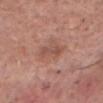Clinical impression: Imaged during a routine full-body skin examination; the lesion was not biopsied and no histopathology is available. Context: On the chest. The recorded lesion diameter is about 3 mm. A roughly 15 mm field-of-view crop from a total-body skin photograph. A male subject, in their mid- to late 60s.